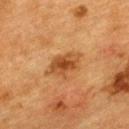site: the upper back
image: total-body-photography crop, ~15 mm field of view
subject: female, aged around 55
illumination: cross-polarized